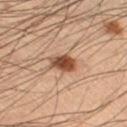Impression: Captured during whole-body skin photography for melanoma surveillance; the lesion was not biopsied. Acquisition and patient details: A region of skin cropped from a whole-body photographic capture, roughly 15 mm wide. A male subject aged 53–57. Automated image analysis of the tile measured a border-irregularity index near 1.5/10, a color-variation rating of about 4.5/10, and radial color variation of about 1.5. The software also gave a detector confidence of about 100 out of 100 that the crop contains a lesion. Imaged with cross-polarized lighting. Approximately 3 mm at its widest. Located on the left lower leg.